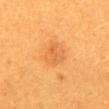| key | value |
|---|---|
| follow-up | catalogued during a skin exam; not biopsied |
| lesion diameter | ~3.5 mm (longest diameter) |
| subject | female, about 30 years old |
| TBP lesion metrics | a lesion color around L≈59 a*≈27 b*≈46 in CIELAB, roughly 7 lightness units darker than nearby skin, and a normalized border contrast of about 4.5; a classifier nevus-likeness of about 35/100 and a lesion-detection confidence of about 100/100 |
| anatomic site | the mid back |
| image source | 15 mm crop, total-body photography |